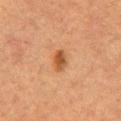biopsy_status: not biopsied; imaged during a skin examination
automated_metrics:
  area_mm2_approx: 4.5
  eccentricity: 0.75
  shape_asymmetry: 0.25
  color_variation_0_10: 4.0
  peripheral_color_asymmetry: 1.5
site: chest
lighting: cross-polarized
image:
  source: total-body photography crop
  field_of_view_mm: 15
lesion_size:
  long_diameter_mm_approx: 2.5
patient:
  sex: female
  age_approx: 70